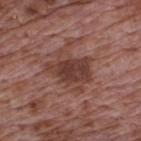{
  "biopsy_status": "not biopsied; imaged during a skin examination",
  "patient": {
    "sex": "male",
    "age_approx": 70
  },
  "site": "upper back",
  "image": {
    "source": "total-body photography crop",
    "field_of_view_mm": 15
  }
}